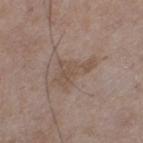notes — catalogued during a skin exam; not biopsied | size — about 4.5 mm | patient — male, in their 50s | site — the left thigh | illumination — white-light | automated lesion analysis — a mean CIELAB color near L≈50 a*≈14 b*≈25, a lesion–skin lightness drop of about 7, and a lesion-to-skin contrast of about 5.5 (normalized; higher = more distinct) | image — ~15 mm crop, total-body skin-cancer survey.